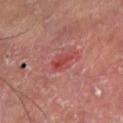{
  "biopsy_status": "not biopsied; imaged during a skin examination",
  "image": {
    "source": "total-body photography crop",
    "field_of_view_mm": 15
  },
  "patient": {
    "sex": "male",
    "age_approx": 55
  },
  "automated_metrics": {
    "vs_skin_darker_L": 7.0,
    "vs_skin_contrast_norm": 6.5
  },
  "lesion_size": {
    "long_diameter_mm_approx": 3.0
  },
  "lighting": "cross-polarized",
  "site": "left lower leg"
}